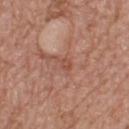{
  "site": "upper back",
  "image": {
    "source": "total-body photography crop",
    "field_of_view_mm": 15
  },
  "patient": {
    "sex": "male",
    "age_approx": 75
  },
  "lighting": "white-light"
}